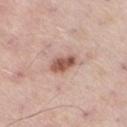Recorded during total-body skin imaging; not selected for excision or biopsy. Cropped from a total-body skin-imaging series; the visible field is about 15 mm. Automated image analysis of the tile measured a footprint of about 5 mm², an outline eccentricity of about 0.75 (0 = round, 1 = elongated), and a shape-asymmetry score of about 0.2 (0 = symmetric). And it measured a mean CIELAB color near L≈55 a*≈23 b*≈27, a lesion–skin lightness drop of about 15, and a lesion-to-skin contrast of about 9.5 (normalized; higher = more distinct). The software also gave a border-irregularity rating of about 2/10 and a within-lesion color-variation index near 4/10. The software also gave a classifier nevus-likeness of about 90/100 and lesion-presence confidence of about 100/100. The patient is a male roughly 55 years of age. Approximately 3 mm at its widest. Located on the right thigh.